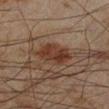| key | value |
|---|---|
| lighting | cross-polarized illumination |
| lesion diameter | about 4 mm |
| imaging modality | total-body-photography crop, ~15 mm field of view |
| subject | male, aged 43 to 47 |
| body site | the leg |